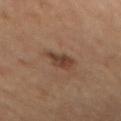- biopsy status — catalogued during a skin exam; not biopsied
- size — ≈3.5 mm
- tile lighting — cross-polarized illumination
- imaging modality — ~15 mm tile from a whole-body skin photo
- subject — female, approximately 60 years of age
- location — the mid back